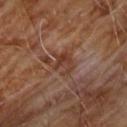<tbp_lesion>
  <biopsy_status>not biopsied; imaged during a skin examination</biopsy_status>
  <automated_metrics>
    <area_mm2_approx>5.5</area_mm2_approx>
    <eccentricity>0.7</eccentricity>
    <shape_asymmetry>0.4</shape_asymmetry>
    <border_irregularity_0_10>6.0</border_irregularity_0_10>
  </automated_metrics>
  <patient>
    <sex>male</sex>
    <age_approx>60</age_approx>
  </patient>
  <lighting>cross-polarized</lighting>
  <image>
    <source>total-body photography crop</source>
    <field_of_view_mm>15</field_of_view_mm>
  </image>
  <site>front of the torso</site>
  <lesion_size>
    <long_diameter_mm_approx>3.5</long_diameter_mm_approx>
  </lesion_size>
</tbp_lesion>